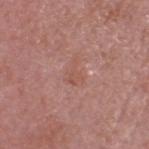Measured at roughly 3 mm in maximum diameter. A male subject, approximately 65 years of age. A region of skin cropped from a whole-body photographic capture, roughly 15 mm wide. From the head or neck.